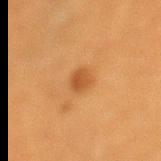Case summary:
• follow-up: catalogued during a skin exam; not biopsied
• illumination: cross-polarized
• lesion diameter: ≈2.5 mm
• image-analysis metrics: a shape eccentricity near 0.7 and a symmetry-axis asymmetry near 0.15; a mean CIELAB color near L≈43 a*≈22 b*≈37, a lesion–skin lightness drop of about 8, and a lesion-to-skin contrast of about 6.5 (normalized; higher = more distinct); a border-irregularity rating of about 1.5/10, a color-variation rating of about 2/10, and a peripheral color-asymmetry measure near 1
• body site: the left lower leg
• subject: female, approximately 40 years of age
• image: total-body-photography crop, ~15 mm field of view